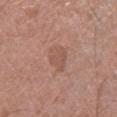Case summary:
• workup — imaged on a skin check; not biopsied
• subject — male, aged 58 to 62
• lighting — white-light
• lesion diameter — ~3 mm (longest diameter)
• automated metrics — an area of roughly 6 mm² and a shape-asymmetry score of about 0.25 (0 = symmetric); a border-irregularity rating of about 3/10 and a within-lesion color-variation index near 1.5/10
• acquisition — total-body-photography crop, ~15 mm field of view
• site — the left lower leg